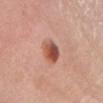workup: total-body-photography surveillance lesion; no biopsy | acquisition: 15 mm crop, total-body photography | subject: female, in their 50s | diameter: ~3 mm (longest diameter) | location: the head or neck | illumination: white-light illumination.